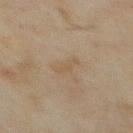  biopsy_status: not biopsied; imaged during a skin examination
  image:
    source: total-body photography crop
    field_of_view_mm: 15
  lesion_size:
    long_diameter_mm_approx: 3.5
  site: chest
  lighting: cross-polarized
  patient:
    sex: male
    age_approx: 70